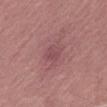Image and clinical context: A 15 mm close-up extracted from a 3D total-body photography capture. Longest diameter approximately 3.5 mm. From the right thigh. This is a white-light tile. A female patient approximately 60 years of age.